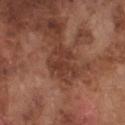<case>
<biopsy_status>not biopsied; imaged during a skin examination</biopsy_status>
<patient>
  <sex>male</sex>
  <age_approx>75</age_approx>
</patient>
<lesion_size>
  <long_diameter_mm_approx>4.5</long_diameter_mm_approx>
</lesion_size>
<automated_metrics>
  <cielab_L>38</cielab_L>
  <cielab_a>23</cielab_a>
  <cielab_b>28</cielab_b>
  <vs_skin_darker_L>8.0</vs_skin_darker_L>
  <vs_skin_contrast_norm>6.5</vs_skin_contrast_norm>
  <border_irregularity_0_10>5.0</border_irregularity_0_10>
  <color_variation_0_10>3.0</color_variation_0_10>
  <peripheral_color_asymmetry>1.0</peripheral_color_asymmetry>
  <nevus_likeness_0_100>0</nevus_likeness_0_100>
  <lesion_detection_confidence_0_100>85</lesion_detection_confidence_0_100>
</automated_metrics>
<site>chest</site>
<image>
  <source>total-body photography crop</source>
  <field_of_view_mm>15</field_of_view_mm>
</image>
</case>